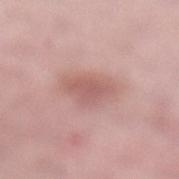No biopsy was performed on this lesion — it was imaged during a full skin examination and was not determined to be concerning. The lesion is on the left lower leg. A 15 mm close-up tile from a total-body photography series done for melanoma screening. The patient is a female aged 63–67.